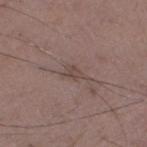Impression: Imaged during a routine full-body skin examination; the lesion was not biopsied and no histopathology is available. Acquisition and patient details: Located on the left thigh. A male subject about 50 years old. A roughly 15 mm field-of-view crop from a total-body skin photograph. This is a white-light tile. The lesion's longest dimension is about 2.5 mm.